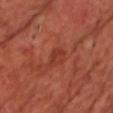follow-up=imaged on a skin check; not biopsied.